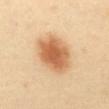Impression: No biopsy was performed on this lesion — it was imaged during a full skin examination and was not determined to be concerning. Acquisition and patient details: Imaged with cross-polarized lighting. A female subject, roughly 40 years of age. The lesion is located on the abdomen. Automated image analysis of the tile measured a lesion area of about 17 mm², an outline eccentricity of about 0.65 (0 = round, 1 = elongated), and two-axis asymmetry of about 0.1. The software also gave a normalized lesion–skin contrast near 9. The software also gave a border-irregularity index near 1.5/10 and internal color variation of about 4.5 on a 0–10 scale. The software also gave a classifier nevus-likeness of about 100/100. The lesion's longest dimension is about 5.5 mm. A region of skin cropped from a whole-body photographic capture, roughly 15 mm wide.